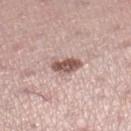  biopsy_status: not biopsied; imaged during a skin examination
  image:
    source: total-body photography crop
    field_of_view_mm: 15
  lighting: white-light
  automated_metrics:
    cielab_L: 55
    cielab_a: 20
    cielab_b: 23
    color_variation_0_10: 3.5
    nevus_likeness_0_100: 85
  patient:
    sex: female
    age_approx: 35
  lesion_size:
    long_diameter_mm_approx: 3.5
  site: right lower leg